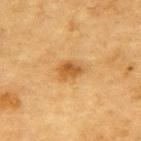Imaged during a routine full-body skin examination; the lesion was not biopsied and no histopathology is available. The lesion is located on the upper back. A male patient, in their mid- to late 80s or older. Approximately 3 mm at its widest. Automated image analysis of the tile measured an outline eccentricity of about 0.7 (0 = round, 1 = elongated) and a shape-asymmetry score of about 0.25 (0 = symmetric). The analysis additionally found a nevus-likeness score of about 85/100 and a lesion-detection confidence of about 100/100. A lesion tile, about 15 mm wide, cut from a 3D total-body photograph.